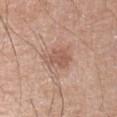biopsy status: no biopsy performed (imaged during a skin exam)
subject: male, about 65 years old
site: the right upper arm
tile lighting: white-light
image: ~15 mm crop, total-body skin-cancer survey
diameter: ~3.5 mm (longest diameter)
image-analysis metrics: a footprint of about 7 mm², an eccentricity of roughly 0.75, and two-axis asymmetry of about 0.3; a mean CIELAB color near L≈56 a*≈21 b*≈28, a lesion–skin lightness drop of about 9, and a lesion-to-skin contrast of about 6 (normalized; higher = more distinct)